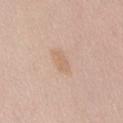Q: Is there a histopathology result?
A: imaged on a skin check; not biopsied
Q: What kind of image is this?
A: ~15 mm crop, total-body skin-cancer survey
Q: What are the patient's age and sex?
A: male, approximately 55 years of age
Q: Lesion location?
A: the abdomen